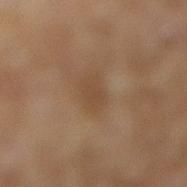Impression: No biopsy was performed on this lesion — it was imaged during a full skin examination and was not determined to be concerning. Clinical summary: Cropped from a whole-body photographic skin survey; the tile spans about 15 mm. The lesion is located on the right lower leg. Measured at roughly 4 mm in maximum diameter. A female subject aged 58–62. Captured under cross-polarized illumination.